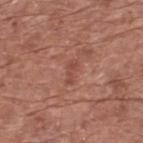workup: imaged on a skin check; not biopsied
patient: male, roughly 75 years of age
acquisition: total-body-photography crop, ~15 mm field of view
anatomic site: the upper back
illumination: white-light illumination
automated metrics: an area of roughly 3 mm², an eccentricity of roughly 0.9, and a symmetry-axis asymmetry near 0.3; a mean CIELAB color near L≈48 a*≈25 b*≈28, a lesion–skin lightness drop of about 7, and a lesion-to-skin contrast of about 5.5 (normalized; higher = more distinct); an automated nevus-likeness rating near 0 out of 100 and a detector confidence of about 100 out of 100 that the crop contains a lesion
size: about 3 mm